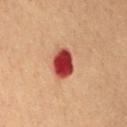Impression: Part of a total-body skin-imaging series; this lesion was reviewed on a skin check and was not flagged for biopsy. Background: A 15 mm close-up tile from a total-body photography series done for melanoma screening. The patient is a female aged approximately 80. On the mid back. Captured under cross-polarized illumination. The lesion's longest dimension is about 3.5 mm.